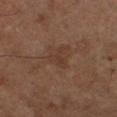biopsy status=catalogued during a skin exam; not biopsied
subject=male, in their mid-60s
body site=the left lower leg
image source=~15 mm tile from a whole-body skin photo
image-analysis metrics=border irregularity of about 6 on a 0–10 scale and internal color variation of about 0.5 on a 0–10 scale; an automated nevus-likeness rating near 0 out of 100 and lesion-presence confidence of about 100/100
diameter=about 3 mm
illumination=cross-polarized